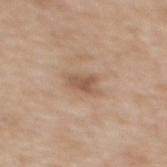Captured during whole-body skin photography for melanoma surveillance; the lesion was not biopsied. About 3 mm across. A 15 mm crop from a total-body photograph taken for skin-cancer surveillance. On the back. The patient is a female aged 53–57.